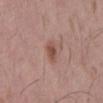Recorded during total-body skin imaging; not selected for excision or biopsy. The lesion is on the mid back. The patient is a male roughly 55 years of age. Measured at roughly 2.5 mm in maximum diameter. This is a white-light tile. A 15 mm close-up extracted from a 3D total-body photography capture.